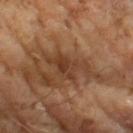No biopsy was performed on this lesion — it was imaged during a full skin examination and was not determined to be concerning.